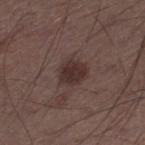workup: total-body-photography surveillance lesion; no biopsy | acquisition: total-body-photography crop, ~15 mm field of view | diameter: about 3.5 mm | image-analysis metrics: a mean CIELAB color near L≈31 a*≈16 b*≈17 and a lesion-to-skin contrast of about 9 (normalized; higher = more distinct); an automated nevus-likeness rating near 65 out of 100 and a detector confidence of about 100 out of 100 that the crop contains a lesion | subject: male, in their mid-50s | site: the right lower leg | lighting: white-light illumination.